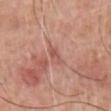Recorded during total-body skin imaging; not selected for excision or biopsy.
This image is a 15 mm lesion crop taken from a total-body photograph.
From the chest.
The subject is a male about 60 years old.